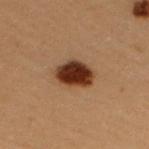This lesion was catalogued during total-body skin photography and was not selected for biopsy.
The tile uses cross-polarized illumination.
On the left arm.
A roughly 15 mm field-of-view crop from a total-body skin photograph.
The subject is a female roughly 30 years of age.